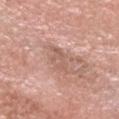workup: no biopsy performed (imaged during a skin exam).